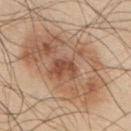Part of a total-body skin-imaging series; this lesion was reviewed on a skin check and was not flagged for biopsy. A male subject in their mid-40s. The lesion is located on the upper back. A 15 mm close-up tile from a total-body photography series done for melanoma screening. Imaged with white-light lighting. Approximately 11.5 mm at its widest.